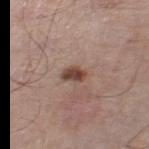notes = total-body-photography surveillance lesion; no biopsy
lighting = white-light illumination
diameter = about 2.5 mm
image = ~15 mm crop, total-body skin-cancer survey
subject = male, about 70 years old
site = the right thigh